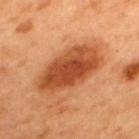biopsy status: total-body-photography surveillance lesion; no biopsy
subject: male, about 50 years old
body site: the upper back
image: ~15 mm tile from a whole-body skin photo
TBP lesion metrics: a mean CIELAB color near L≈51 a*≈30 b*≈41, about 15 CIELAB-L* units darker than the surrounding skin, and a lesion-to-skin contrast of about 10 (normalized; higher = more distinct); a classifier nevus-likeness of about 90/100 and a lesion-detection confidence of about 100/100
tile lighting: cross-polarized illumination
lesion size: about 8.5 mm